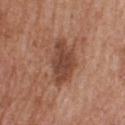Captured during whole-body skin photography for melanoma surveillance; the lesion was not biopsied. The patient is a male aged approximately 60. From the upper back. A region of skin cropped from a whole-body photographic capture, roughly 15 mm wide. About 5.5 mm across. Imaged with white-light lighting.